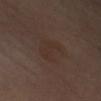Notes:
– notes · imaged on a skin check; not biopsied
– location · the right upper arm
– acquisition · ~15 mm tile from a whole-body skin photo
– lesion size · ≈4 mm
– patient · female, aged 58 to 62
– automated lesion analysis · a border-irregularity rating of about 4/10 and peripheral color asymmetry of about 0.5
– tile lighting · cross-polarized illumination